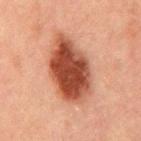Case summary:
- biopsy status — imaged on a skin check; not biopsied
- patient — male, aged 48–52
- body site — the back
- size — ≈7.5 mm
- tile lighting — cross-polarized
- imaging modality — ~15 mm crop, total-body skin-cancer survey